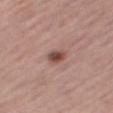Assessment: The lesion was photographed on a routine skin check and not biopsied; there is no pathology result. Image and clinical context: This is a white-light tile. A close-up tile cropped from a whole-body skin photograph, about 15 mm across. An algorithmic analysis of the crop reported a lesion area of about 4 mm² and a shape-asymmetry score of about 0.2 (0 = symmetric). The software also gave a lesion color around L≈48 a*≈21 b*≈24 in CIELAB, about 13 CIELAB-L* units darker than the surrounding skin, and a normalized border contrast of about 9. And it measured border irregularity of about 1.5 on a 0–10 scale, a within-lesion color-variation index near 6.5/10, and radial color variation of about 2.5. It also reported a classifier nevus-likeness of about 90/100. The lesion's longest dimension is about 2.5 mm. On the right thigh. A male patient, aged around 75.